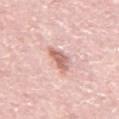Assessment: Recorded during total-body skin imaging; not selected for excision or biopsy. Context: The tile uses white-light illumination. A male subject in their mid- to late 70s. A roughly 15 mm field-of-view crop from a total-body skin photograph. The lesion-visualizer software estimated an outline eccentricity of about 0.75 (0 = round, 1 = elongated) and two-axis asymmetry of about 0.3. And it measured a border-irregularity index near 3.5/10 and radial color variation of about 1. The analysis additionally found a nevus-likeness score of about 60/100. On the back.